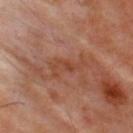Impression:
This lesion was catalogued during total-body skin photography and was not selected for biopsy.
Acquisition and patient details:
A male subject aged 48–52. About 4.5 mm across. Cropped from a whole-body photographic skin survey; the tile spans about 15 mm. The lesion is located on the upper back.